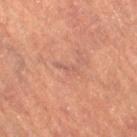Case summary:
– size · ≈3 mm
– imaging modality · total-body-photography crop, ~15 mm field of view
– anatomic site · the leg
– patient · female, approximately 70 years of age
– automated metrics · a symmetry-axis asymmetry near 0.45
– tile lighting · cross-polarized